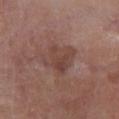This lesion was catalogued during total-body skin photography and was not selected for biopsy. Imaged with white-light lighting. A 15 mm crop from a total-body photograph taken for skin-cancer surveillance. Measured at roughly 4.5 mm in maximum diameter. From the left lower leg. A male patient, in their 70s.